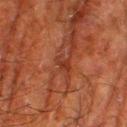Acquisition and patient details:
Located on the right thigh. The lesion's longest dimension is about 2.5 mm. This is a cross-polarized tile. The total-body-photography lesion software estimated two-axis asymmetry of about 0.25. The software also gave an average lesion color of about L≈29 a*≈24 b*≈27 (CIELAB) and a lesion–skin lightness drop of about 6. Cropped from a whole-body photographic skin survey; the tile spans about 15 mm. A male patient roughly 80 years of age.